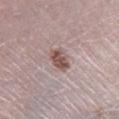| field | value |
|---|---|
| notes | no biopsy performed (imaged during a skin exam) |
| lesion size | ≈3.5 mm |
| patient | male, aged around 50 |
| imaging modality | ~15 mm tile from a whole-body skin photo |
| tile lighting | white-light |
| location | the right lower leg |